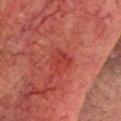Captured during whole-body skin photography for melanoma surveillance; the lesion was not biopsied. Captured under cross-polarized illumination. The lesion's longest dimension is about 3 mm. The lesion-visualizer software estimated a lesion color around L≈32 a*≈30 b*≈24 in CIELAB and a normalized border contrast of about 5. Located on the head or neck. A male patient about 60 years old. A 15 mm close-up extracted from a 3D total-body photography capture.